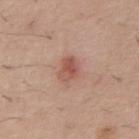Assessment:
The lesion was tiled from a total-body skin photograph and was not biopsied.
Clinical summary:
The lesion is located on the abdomen. Automated tile analysis of the lesion measured a lesion area of about 5 mm², a shape eccentricity near 0.65, and a symmetry-axis asymmetry near 0.3. The analysis additionally found a mean CIELAB color near L≈54 a*≈24 b*≈28 and roughly 10 lightness units darker than nearby skin. The analysis additionally found a classifier nevus-likeness of about 50/100 and a detector confidence of about 100 out of 100 that the crop contains a lesion. A region of skin cropped from a whole-body photographic capture, roughly 15 mm wide. A male patient aged 58 to 62. The tile uses white-light illumination.